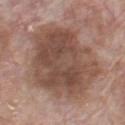The lesion was photographed on a routine skin check and not biopsied; there is no pathology result. The lesion-visualizer software estimated a lesion area of about 55 mm² and a shape eccentricity near 0.6. And it measured a border-irregularity rating of about 2.5/10 and internal color variation of about 5.5 on a 0–10 scale. From the chest. A male subject, aged 63 to 67. About 10 mm across. A 15 mm close-up tile from a total-body photography series done for melanoma screening.